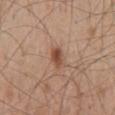biopsy status — total-body-photography surveillance lesion; no biopsy | site — the back | automated metrics — an average lesion color of about L≈50 a*≈21 b*≈30 (CIELAB), about 10 CIELAB-L* units darker than the surrounding skin, and a normalized border contrast of about 7.5; a border-irregularity index near 2.5/10, a within-lesion color-variation index near 5/10, and radial color variation of about 1.5; a classifier nevus-likeness of about 90/100 and a lesion-detection confidence of about 100/100 | subject — male, in their 50s | image source — total-body-photography crop, ~15 mm field of view | tile lighting — white-light | lesion size — ~3 mm (longest diameter).